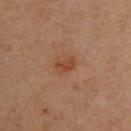notes: no biopsy performed (imaged during a skin exam); subject: female, in their 40s; acquisition: ~15 mm crop, total-body skin-cancer survey; size: ~2.5 mm (longest diameter); anatomic site: the upper back.